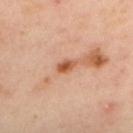Findings:
– workup — imaged on a skin check; not biopsied
– subject — female, aged around 40
– body site — the upper back
– image — total-body-photography crop, ~15 mm field of view
– lighting — cross-polarized
– size — about 2.5 mm